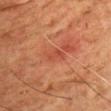{"biopsy_status": "not biopsied; imaged during a skin examination", "lighting": "cross-polarized", "lesion_size": {"long_diameter_mm_approx": 2.5}, "image": {"source": "total-body photography crop", "field_of_view_mm": 15}, "automated_metrics": {"cielab_L": 39, "cielab_a": 27, "cielab_b": 29, "vs_skin_darker_L": 6.0, "vs_skin_contrast_norm": 5.0}, "patient": {"sex": "male", "age_approx": 65}, "site": "chest"}